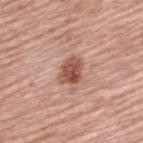  biopsy_status: not biopsied; imaged during a skin examination
  patient:
    sex: male
    age_approx: 80
  image:
    source: total-body photography crop
    field_of_view_mm: 15
  lighting: white-light
  lesion_size:
    long_diameter_mm_approx: 3.5
  site: upper back
  automated_metrics:
    vs_skin_darker_L: 13.0
    vs_skin_contrast_norm: 9.0
    border_irregularity_0_10: 2.5
    color_variation_0_10: 5.0
    peripheral_color_asymmetry: 2.0
    nevus_likeness_0_100: 90
    lesion_detection_confidence_0_100: 100